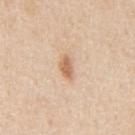Assessment: Imaged during a routine full-body skin examination; the lesion was not biopsied and no histopathology is available. Context: A male subject, about 60 years old. A region of skin cropped from a whole-body photographic capture, roughly 15 mm wide. The lesion is on the mid back.